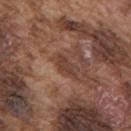Impression:
No biopsy was performed on this lesion — it was imaged during a full skin examination and was not determined to be concerning.
Background:
Cropped from a total-body skin-imaging series; the visible field is about 15 mm. The lesion is on the upper back. A male subject, in their mid-70s.